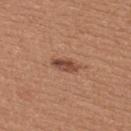This lesion was catalogued during total-body skin photography and was not selected for biopsy.
Captured under white-light illumination.
A close-up tile cropped from a whole-body skin photograph, about 15 mm across.
A female patient aged 53 to 57.
On the upper back.